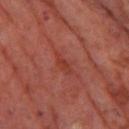Clinical impression:
Recorded during total-body skin imaging; not selected for excision or biopsy.
Acquisition and patient details:
On the left thigh. A male patient roughly 70 years of age. This image is a 15 mm lesion crop taken from a total-body photograph. The recorded lesion diameter is about 3 mm.